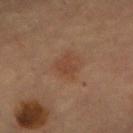{
  "biopsy_status": "not biopsied; imaged during a skin examination",
  "site": "abdomen",
  "patient": {
    "sex": "female",
    "age_approx": 60
  },
  "image": {
    "source": "total-body photography crop",
    "field_of_view_mm": 15
  },
  "lesion_size": {
    "long_diameter_mm_approx": 3.0
  }
}